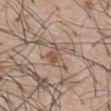Q: Was this lesion biopsied?
A: imaged on a skin check; not biopsied
Q: What is the anatomic site?
A: the mid back
Q: What is the lesion's diameter?
A: ~4.5 mm (longest diameter)
Q: What are the patient's age and sex?
A: male, aged 53 to 57
Q: What lighting was used for the tile?
A: white-light
Q: How was this image acquired?
A: total-body-photography crop, ~15 mm field of view